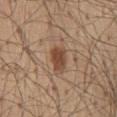<case>
<biopsy_status>not biopsied; imaged during a skin examination</biopsy_status>
</case>